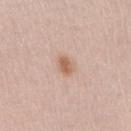Q: Was this lesion biopsied?
A: total-body-photography surveillance lesion; no biopsy
Q: Automated lesion metrics?
A: a shape eccentricity near 0.65; a mean CIELAB color near L≈62 a*≈20 b*≈30, a lesion–skin lightness drop of about 10, and a lesion-to-skin contrast of about 7.5 (normalized; higher = more distinct); border irregularity of about 2.5 on a 0–10 scale and internal color variation of about 2.5 on a 0–10 scale; an automated nevus-likeness rating near 85 out of 100
Q: What kind of image is this?
A: 15 mm crop, total-body photography
Q: Patient demographics?
A: female, aged 38 to 42
Q: Where on the body is the lesion?
A: the right upper arm
Q: What lighting was used for the tile?
A: white-light